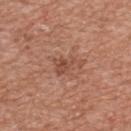Impression: This lesion was catalogued during total-body skin photography and was not selected for biopsy. Image and clinical context: The lesion-visualizer software estimated a footprint of about 5 mm² and two-axis asymmetry of about 0.65. The analysis additionally found a lesion–skin lightness drop of about 9 and a normalized border contrast of about 6.5. The software also gave a color-variation rating of about 2.5/10 and radial color variation of about 1. The software also gave lesion-presence confidence of about 100/100. From the front of the torso. This is a white-light tile. The recorded lesion diameter is about 3.5 mm. A roughly 15 mm field-of-view crop from a total-body skin photograph. The patient is a male in their mid- to late 40s.